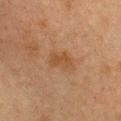Part of a total-body skin-imaging series; this lesion was reviewed on a skin check and was not flagged for biopsy. From the chest. Imaged with cross-polarized lighting. A lesion tile, about 15 mm wide, cut from a 3D total-body photograph. The subject is a female aged around 40.